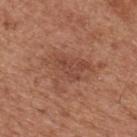| field | value |
|---|---|
| notes | imaged on a skin check; not biopsied |
| anatomic site | the upper back |
| image source | ~15 mm crop, total-body skin-cancer survey |
| tile lighting | white-light illumination |
| subject | male, in their mid- to late 60s |